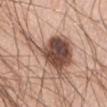Assessment: Imaged during a routine full-body skin examination; the lesion was not biopsied and no histopathology is available. Image and clinical context: The lesion is on the front of the torso. A region of skin cropped from a whole-body photographic capture, roughly 15 mm wide. A female patient, aged 68 to 72.